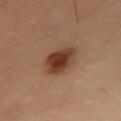Cropped from a whole-body photographic skin survey; the tile spans about 15 mm. The recorded lesion diameter is about 3.5 mm. The lesion is on the front of the torso. This is a cross-polarized tile. A female subject, approximately 60 years of age.